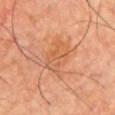Captured during whole-body skin photography for melanoma surveillance; the lesion was not biopsied. The lesion's longest dimension is about 4 mm. Cropped from a total-body skin-imaging series; the visible field is about 15 mm. The lesion is located on the chest. A male subject, in their mid-60s.